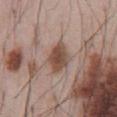Impression: This lesion was catalogued during total-body skin photography and was not selected for biopsy. Acquisition and patient details: Imaged with white-light lighting. The patient is a male aged 53 to 57. A region of skin cropped from a whole-body photographic capture, roughly 15 mm wide. On the abdomen. Approximately 3.5 mm at its widest.